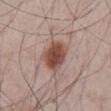The lesion was photographed on a routine skin check and not biopsied; there is no pathology result. A region of skin cropped from a whole-body photographic capture, roughly 15 mm wide. The total-body-photography lesion software estimated roughly 14 lightness units darker than nearby skin and a normalized lesion–skin contrast near 10.5. And it measured a detector confidence of about 100 out of 100 that the crop contains a lesion. A male patient aged 63–67. Approximately 4.5 mm at its widest. This is a white-light tile. Located on the abdomen.